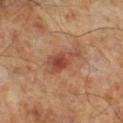notes: no biopsy performed (imaged during a skin exam) | lighting: cross-polarized illumination | image source: 15 mm crop, total-body photography | subject: male, roughly 65 years of age | automated metrics: a shape eccentricity near 0.9 and two-axis asymmetry of about 0.4; a mean CIELAB color near L≈45 a*≈24 b*≈30, about 10 CIELAB-L* units darker than the surrounding skin, and a lesion-to-skin contrast of about 7.5 (normalized; higher = more distinct); a nevus-likeness score of about 75/100 and a lesion-detection confidence of about 100/100.